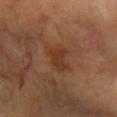Captured during whole-body skin photography for melanoma surveillance; the lesion was not biopsied. Automated tile analysis of the lesion measured two-axis asymmetry of about 0.3. It also reported a border-irregularity index near 3.5/10, a color-variation rating of about 2/10, and radial color variation of about 1. And it measured an automated nevus-likeness rating near 0 out of 100. The lesion is located on the arm. Measured at roughly 3.5 mm in maximum diameter. The tile uses cross-polarized illumination. A region of skin cropped from a whole-body photographic capture, roughly 15 mm wide. A female patient about 70 years old.